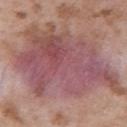Assessment: Captured during whole-body skin photography for melanoma surveillance; the lesion was not biopsied. Clinical summary: The patient is a male in their 50s. Located on the right upper arm. The tile uses white-light illumination. Cropped from a whole-body photographic skin survey; the tile spans about 15 mm. The lesion's longest dimension is about 13 mm.